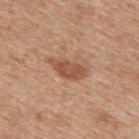Acquisition and patient details: A male patient in their mid-60s. From the upper back. The tile uses white-light illumination. This image is a 15 mm lesion crop taken from a total-body photograph. The lesion's longest dimension is about 4.5 mm.